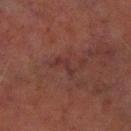  biopsy_status: not biopsied; imaged during a skin examination
  patient:
    sex: male
    age_approx: 70
  image:
    source: total-body photography crop
    field_of_view_mm: 15
  site: left lower leg